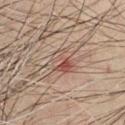The lesion is on the chest. Longest diameter approximately 3.5 mm. Automated image analysis of the tile measured a shape eccentricity near 0.7 and a symmetry-axis asymmetry near 0.4. The software also gave border irregularity of about 5.5 on a 0–10 scale, a within-lesion color-variation index near 6.5/10, and radial color variation of about 2. And it measured a nevus-likeness score of about 20/100 and a detector confidence of about 100 out of 100 that the crop contains a lesion. Cropped from a whole-body photographic skin survey; the tile spans about 15 mm. This is a cross-polarized tile. The subject is a male roughly 60 years of age.